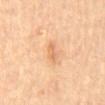Notes:
• workup — imaged on a skin check; not biopsied
• anatomic site — the mid back
• subject — male, aged 83 or over
• image source — total-body-photography crop, ~15 mm field of view